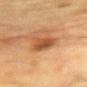workup=no biopsy performed (imaged during a skin exam)
patient=male, in their mid-80s or older
image=~15 mm tile from a whole-body skin photo
size=≈3 mm
site=the chest
illumination=cross-polarized illumination